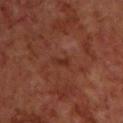Clinical impression:
No biopsy was performed on this lesion — it was imaged during a full skin examination and was not determined to be concerning.
Acquisition and patient details:
The subject is a male roughly 70 years of age. Captured under cross-polarized illumination. The lesion is located on the upper back. Longest diameter approximately 2.5 mm. A roughly 15 mm field-of-view crop from a total-body skin photograph.